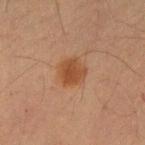Q: Was a biopsy performed?
A: no biopsy performed (imaged during a skin exam)
Q: What kind of image is this?
A: 15 mm crop, total-body photography
Q: What are the patient's age and sex?
A: male, roughly 65 years of age
Q: How was the tile lit?
A: cross-polarized
Q: Automated lesion metrics?
A: a lesion area of about 6.5 mm², an outline eccentricity of about 0.3 (0 = round, 1 = elongated), and a symmetry-axis asymmetry near 0.2; a mean CIELAB color near L≈39 a*≈20 b*≈30, a lesion–skin lightness drop of about 8, and a normalized border contrast of about 8; a border-irregularity index near 2/10, a color-variation rating of about 2/10, and radial color variation of about 1
Q: Where on the body is the lesion?
A: the right forearm
Q: How large is the lesion?
A: ≈3 mm